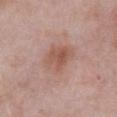Imaged during a routine full-body skin examination; the lesion was not biopsied and no histopathology is available.
Longest diameter approximately 4.5 mm.
The lesion-visualizer software estimated a lesion area of about 12 mm² and an outline eccentricity of about 0.55 (0 = round, 1 = elongated). The analysis additionally found a lesion color around L≈55 a*≈21 b*≈26 in CIELAB, a lesion–skin lightness drop of about 8, and a normalized border contrast of about 6. And it measured an automated nevus-likeness rating near 40 out of 100 and a detector confidence of about 100 out of 100 that the crop contains a lesion.
A male patient, aged around 55.
Captured under white-light illumination.
From the abdomen.
A 15 mm close-up extracted from a 3D total-body photography capture.